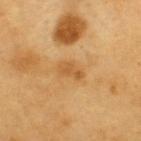The lesion was photographed on a routine skin check and not biopsied; there is no pathology result. The lesion-visualizer software estimated an average lesion color of about L≈56 a*≈21 b*≈44 (CIELAB), about 8 CIELAB-L* units darker than the surrounding skin, and a normalized lesion–skin contrast near 5.5. The software also gave a within-lesion color-variation index near 2/10 and peripheral color asymmetry of about 0.5. The software also gave a classifier nevus-likeness of about 0/100 and a lesion-detection confidence of about 100/100. The subject is a male aged 58–62. A roughly 15 mm field-of-view crop from a total-body skin photograph. Approximately 3 mm at its widest. From the upper back. Imaged with cross-polarized lighting.